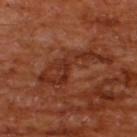Findings:
– workup — total-body-photography surveillance lesion; no biopsy
– TBP lesion metrics — a mean CIELAB color near L≈31 a*≈25 b*≈30 and a normalized border contrast of about 6.5; a classifier nevus-likeness of about 0/100 and a lesion-detection confidence of about 65/100
– location — the upper back
– subject — male, roughly 65 years of age
– image source — total-body-photography crop, ~15 mm field of view
– lesion size — ~9.5 mm (longest diameter)